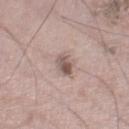Case summary:
- workup · catalogued during a skin exam; not biopsied
- patient · male, aged around 65
- location · the right thigh
- image · ~15 mm tile from a whole-body skin photo